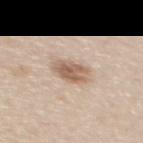automated metrics: a lesion area of about 8 mm², an eccentricity of roughly 0.7, and two-axis asymmetry of about 0.15; a classifier nevus-likeness of about 90/100 and lesion-presence confidence of about 100/100 | tile lighting: white-light illumination | lesion size: ≈4 mm | subject: female, aged 43 to 47 | image source: total-body-photography crop, ~15 mm field of view | body site: the upper back.